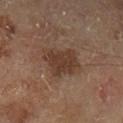Acquisition and patient details: A 15 mm close-up tile from a total-body photography series done for melanoma screening. The lesion is located on the left lower leg. This is a cross-polarized tile. The recorded lesion diameter is about 4 mm. A male patient about 70 years old.